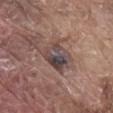subject: male, approximately 80 years of age
lesion diameter: ≈4 mm
body site: the abdomen
automated lesion analysis: a lesion area of about 11 mm², an outline eccentricity of about 0.55 (0 = round, 1 = elongated), and two-axis asymmetry of about 0.2; a mean CIELAB color near L≈43 a*≈14 b*≈17; a nevus-likeness score of about 0/100
tile lighting: white-light
acquisition: ~15 mm tile from a whole-body skin photo